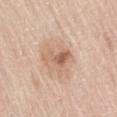Part of a total-body skin-imaging series; this lesion was reviewed on a skin check and was not flagged for biopsy.
Imaged with white-light lighting.
From the right thigh.
The patient is a female aged approximately 65.
A roughly 15 mm field-of-view crop from a total-body skin photograph.